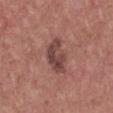This lesion was catalogued during total-body skin photography and was not selected for biopsy. A lesion tile, about 15 mm wide, cut from a 3D total-body photograph. A female patient aged 43 to 47. Located on the chest. Imaged with white-light lighting.